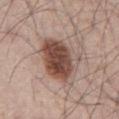Assessment: Part of a total-body skin-imaging series; this lesion was reviewed on a skin check and was not flagged for biopsy. Context: On the abdomen. Cropped from a whole-body photographic skin survey; the tile spans about 15 mm. A male subject, approximately 65 years of age.